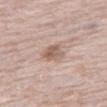Clinical impression:
This lesion was catalogued during total-body skin photography and was not selected for biopsy.
Image and clinical context:
The lesion-visualizer software estimated a lesion area of about 5 mm². The software also gave a lesion color around L≈59 a*≈18 b*≈25 in CIELAB, about 10 CIELAB-L* units darker than the surrounding skin, and a normalized lesion–skin contrast near 7. Captured under white-light illumination. A male patient, aged around 75. The lesion is on the right thigh. This image is a 15 mm lesion crop taken from a total-body photograph.